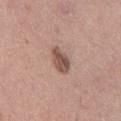Captured during whole-body skin photography for melanoma surveillance; the lesion was not biopsied. The lesion is located on the right thigh. A female subject, about 40 years old. This is a white-light tile. A 15 mm crop from a total-body photograph taken for skin-cancer surveillance.